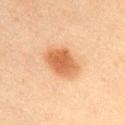{"biopsy_status": "not biopsied; imaged during a skin examination", "site": "chest", "image": {"source": "total-body photography crop", "field_of_view_mm": 15}, "patient": {"sex": "male", "age_approx": 45}, "automated_metrics": {"cielab_L": 51, "cielab_a": 21, "cielab_b": 35, "vs_skin_contrast_norm": 8.5, "border_irregularity_0_10": 1.5, "color_variation_0_10": 3.0, "peripheral_color_asymmetry": 1.0}, "lighting": "cross-polarized", "lesion_size": {"long_diameter_mm_approx": 4.5}}